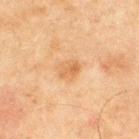Captured during whole-body skin photography for melanoma surveillance; the lesion was not biopsied. Located on the front of the torso. Cropped from a whole-body photographic skin survey; the tile spans about 15 mm. A male patient aged around 65.